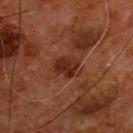This lesion was catalogued during total-body skin photography and was not selected for biopsy.
A 15 mm crop from a total-body photograph taken for skin-cancer surveillance.
A male patient in their mid- to late 50s.
This is a cross-polarized tile.
About 3 mm across.
The lesion is located on the chest.